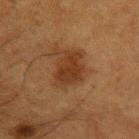Impression:
No biopsy was performed on this lesion — it was imaged during a full skin examination and was not determined to be concerning.
Image and clinical context:
Cropped from a total-body skin-imaging series; the visible field is about 15 mm. A male patient in their 50s. From the left upper arm.